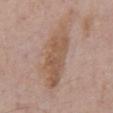Part of a total-body skin-imaging series; this lesion was reviewed on a skin check and was not flagged for biopsy. A close-up tile cropped from a whole-body skin photograph, about 15 mm across. A male patient, aged 68 to 72. The lesion is located on the abdomen.